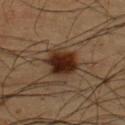The lesion was tiled from a total-body skin photograph and was not biopsied. The lesion is on the back. The patient is a male aged approximately 50. Cropped from a total-body skin-imaging series; the visible field is about 15 mm. The tile uses cross-polarized illumination. Approximately 4 mm at its widest.